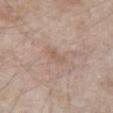Clinical impression: Recorded during total-body skin imaging; not selected for excision or biopsy. Background: A roughly 15 mm field-of-view crop from a total-body skin photograph. The lesion-visualizer software estimated an eccentricity of roughly 0.9 and a symmetry-axis asymmetry near 0.25. And it measured a lesion color around L≈57 a*≈17 b*≈27 in CIELAB, about 6 CIELAB-L* units darker than the surrounding skin, and a normalized border contrast of about 5. The analysis additionally found border irregularity of about 3 on a 0–10 scale. The analysis additionally found a nevus-likeness score of about 0/100. The patient is a male approximately 65 years of age. The lesion is on the abdomen. Captured under white-light illumination. Longest diameter approximately 3 mm.